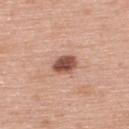Q: Was this lesion biopsied?
A: imaged on a skin check; not biopsied
Q: What kind of image is this?
A: ~15 mm crop, total-body skin-cancer survey
Q: What is the anatomic site?
A: the upper back
Q: What lighting was used for the tile?
A: white-light
Q: Automated lesion metrics?
A: a lesion area of about 6.5 mm², a shape eccentricity near 0.6, and a shape-asymmetry score of about 0.15 (0 = symmetric); a nevus-likeness score of about 95/100 and lesion-presence confidence of about 100/100
Q: What are the patient's age and sex?
A: male, about 60 years old
Q: Lesion size?
A: ≈3 mm